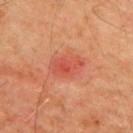{"biopsy_status": "not biopsied; imaged during a skin examination", "lighting": "cross-polarized", "image": {"source": "total-body photography crop", "field_of_view_mm": 15}, "site": "upper back", "lesion_size": {"long_diameter_mm_approx": 4.0}, "automated_metrics": {"area_mm2_approx": 9.0, "eccentricity": 0.7, "shape_asymmetry": 0.15, "cielab_L": 54, "cielab_a": 35, "cielab_b": 35, "vs_skin_darker_L": 8.0, "vs_skin_contrast_norm": 5.5, "nevus_likeness_0_100": 0}, "patient": {"sex": "male", "age_approx": 65}}